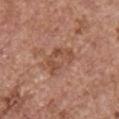notes: catalogued during a skin exam; not biopsied | image: ~15 mm tile from a whole-body skin photo | image-analysis metrics: a border-irregularity index near 4/10 and peripheral color asymmetry of about 1; a nevus-likeness score of about 5/100 and lesion-presence confidence of about 100/100 | lesion diameter: ≈4.5 mm | patient: female, in their mid-60s | lighting: white-light illumination | site: the chest.